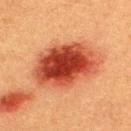Case summary:
* notes: total-body-photography surveillance lesion; no biopsy
* patient: male, about 40 years old
* tile lighting: cross-polarized illumination
* acquisition: total-body-photography crop, ~15 mm field of view
* automated lesion analysis: a mean CIELAB color near L≈40 a*≈31 b*≈33 and a lesion–skin lightness drop of about 16; border irregularity of about 1.5 on a 0–10 scale, a within-lesion color-variation index near 7.5/10, and a peripheral color-asymmetry measure near 2
* location: the back
* lesion size: ≈8 mm